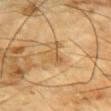| feature | finding |
|---|---|
| notes | imaged on a skin check; not biopsied |
| subject | male, aged around 85 or older |
| illumination | cross-polarized |
| imaging modality | total-body-photography crop, ~15 mm field of view |
| lesion size | ≈4 mm |
| anatomic site | the chest |